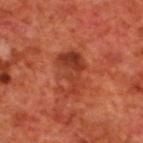{
  "biopsy_status": "not biopsied; imaged during a skin examination",
  "site": "upper back",
  "image": {
    "source": "total-body photography crop",
    "field_of_view_mm": 15
  },
  "patient": {
    "sex": "male",
    "age_approx": 70
  }
}